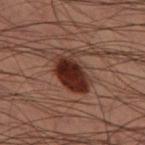Q: What is the imaging modality?
A: 15 mm crop, total-body photography
Q: Patient demographics?
A: male, in their mid-50s
Q: Where on the body is the lesion?
A: the left thigh
Q: What lighting was used for the tile?
A: cross-polarized illumination
Q: What is the lesion's diameter?
A: ≈5 mm